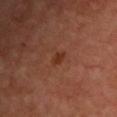Case summary:
– image · 15 mm crop, total-body photography
– lighting · cross-polarized illumination
– patient · male, aged approximately 65
– automated lesion analysis · peripheral color asymmetry of about 0.5; a detector confidence of about 100 out of 100 that the crop contains a lesion
– body site · the chest
– size · ≈2 mm